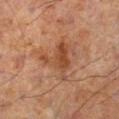tile lighting — cross-polarized illumination | subject — male, roughly 70 years of age | image-analysis metrics — an outline eccentricity of about 0.5 (0 = round, 1 = elongated); about 8 CIELAB-L* units darker than the surrounding skin and a lesion-to-skin contrast of about 7 (normalized; higher = more distinct); a lesion-detection confidence of about 100/100 | location — the left lower leg | image — ~15 mm crop, total-body skin-cancer survey.